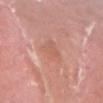This lesion was catalogued during total-body skin photography and was not selected for biopsy. A 15 mm close-up extracted from a 3D total-body photography capture. The recorded lesion diameter is about 3.5 mm. On the left lower leg. The total-body-photography lesion software estimated an outline eccentricity of about 0.75 (0 = round, 1 = elongated). The analysis additionally found an average lesion color of about L≈60 a*≈24 b*≈29 (CIELAB), roughly 6 lightness units darker than nearby skin, and a lesion-to-skin contrast of about 4.5 (normalized; higher = more distinct). The subject is a male approximately 40 years of age.